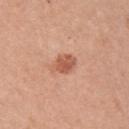Clinical impression: The lesion was tiled from a total-body skin photograph and was not biopsied. Clinical summary: A female patient, roughly 60 years of age. The tile uses white-light illumination. A roughly 15 mm field-of-view crop from a total-body skin photograph. Located on the left upper arm.